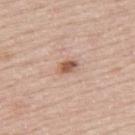The lesion was tiled from a total-body skin photograph and was not biopsied. This image is a 15 mm lesion crop taken from a total-body photograph. Captured under white-light illumination. The lesion is on the upper back. Approximately 2.5 mm at its widest. The subject is a male aged around 50. The total-body-photography lesion software estimated an outline eccentricity of about 0.8 (0 = round, 1 = elongated) and two-axis asymmetry of about 0.3. The analysis additionally found an average lesion color of about L≈56 a*≈21 b*≈30 (CIELAB) and a normalized lesion–skin contrast near 9. And it measured a border-irregularity index near 2.5/10 and radial color variation of about 1.